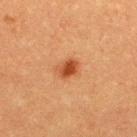Captured during whole-body skin photography for melanoma surveillance; the lesion was not biopsied. A male patient roughly 40 years of age. Imaged with cross-polarized lighting. Longest diameter approximately 2.5 mm. A roughly 15 mm field-of-view crop from a total-body skin photograph. The lesion-visualizer software estimated an area of roughly 4 mm², an outline eccentricity of about 0.65 (0 = round, 1 = elongated), and two-axis asymmetry of about 0.2. It also reported a mean CIELAB color near L≈41 a*≈26 b*≈35 and about 12 CIELAB-L* units darker than the surrounding skin. And it measured border irregularity of about 2 on a 0–10 scale and a within-lesion color-variation index near 2.5/10. The software also gave a nevus-likeness score of about 100/100 and lesion-presence confidence of about 100/100. From the back.